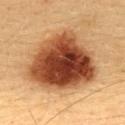Clinical impression:
Part of a total-body skin-imaging series; this lesion was reviewed on a skin check and was not flagged for biopsy.
Image and clinical context:
Imaged with cross-polarized lighting. From the back. A female subject, approximately 30 years of age. A 15 mm close-up tile from a total-body photography series done for melanoma screening. About 9 mm across.